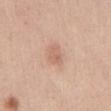{
  "biopsy_status": "not biopsied; imaged during a skin examination",
  "image": {
    "source": "total-body photography crop",
    "field_of_view_mm": 15
  },
  "lesion_size": {
    "long_diameter_mm_approx": 2.5
  },
  "patient": {
    "sex": "female",
    "age_approx": 35
  },
  "site": "abdomen"
}